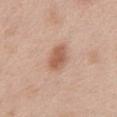• follow-up · total-body-photography surveillance lesion; no biopsy
• image-analysis metrics · a classifier nevus-likeness of about 95/100
• illumination · white-light
• site · the abdomen
• patient · female, approximately 40 years of age
• acquisition · ~15 mm tile from a whole-body skin photo